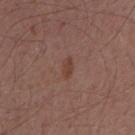The recorded lesion diameter is about 2.5 mm.
The lesion is on the chest.
A 15 mm crop from a total-body photograph taken for skin-cancer surveillance.
The tile uses white-light illumination.
The subject is a male in their mid-50s.